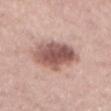Clinical impression: This lesion was catalogued during total-body skin photography and was not selected for biopsy. Clinical summary: Cropped from a whole-body photographic skin survey; the tile spans about 15 mm. This is a white-light tile. The subject is a male aged 63 to 67. From the mid back. Automated image analysis of the tile measured an average lesion color of about L≈54 a*≈21 b*≈23 (CIELAB) and roughly 16 lightness units darker than nearby skin. And it measured internal color variation of about 5 on a 0–10 scale and radial color variation of about 1.5.